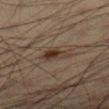Assessment:
Imaged during a routine full-body skin examination; the lesion was not biopsied and no histopathology is available.
Background:
The lesion is on the left lower leg. A male patient aged 33 to 37. This image is a 15 mm lesion crop taken from a total-body photograph. The lesion-visualizer software estimated an eccentricity of roughly 0.8 and two-axis asymmetry of about 0.35. Approximately 3.5 mm at its widest.